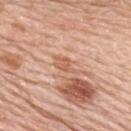Findings:
- body site — the upper back
- image — 15 mm crop, total-body photography
- subject — female, aged 63 to 67